A 15 mm close-up tile from a total-body photography series done for melanoma screening · the tile uses cross-polarized illumination · the lesion is on the arm · the lesion's longest dimension is about 3 mm · a male subject, approximately 65 years of age: 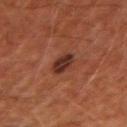Diagnosis:
Histopathology of the biopsied lesion showed a seborrheic keratosis.